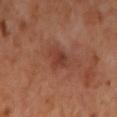Located on the right forearm.
Automated tile analysis of the lesion measured an average lesion color of about L≈40 a*≈26 b*≈30 (CIELAB) and about 8 CIELAB-L* units darker than the surrounding skin. It also reported a border-irregularity index near 3/10, internal color variation of about 3 on a 0–10 scale, and peripheral color asymmetry of about 1. It also reported an automated nevus-likeness rating near 5 out of 100.
The lesion's longest dimension is about 3 mm.
A lesion tile, about 15 mm wide, cut from a 3D total-body photograph.
A female subject aged approximately 50.